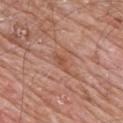{
  "biopsy_status": "not biopsied; imaged during a skin examination",
  "patient": {
    "sex": "male",
    "age_approx": 80
  },
  "site": "mid back",
  "image": {
    "source": "total-body photography crop",
    "field_of_view_mm": 15
  }
}